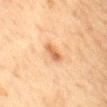Assessment: No biopsy was performed on this lesion — it was imaged during a full skin examination and was not determined to be concerning. Background: A roughly 15 mm field-of-view crop from a total-body skin photograph. Longest diameter approximately 3.5 mm. A female patient, approximately 60 years of age. From the chest. Captured under cross-polarized illumination. Automated tile analysis of the lesion measured a border-irregularity index near 3/10, a color-variation rating of about 3/10, and radial color variation of about 1.